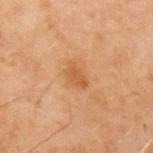workup=no biopsy performed (imaged during a skin exam); acquisition=total-body-photography crop, ~15 mm field of view; subject=male, aged 43 to 47; location=the right upper arm.